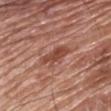follow-up: imaged on a skin check; not biopsied | lesion size: ~5 mm (longest diameter) | subject: male, aged approximately 65 | location: the front of the torso | illumination: white-light illumination | image: 15 mm crop, total-body photography.